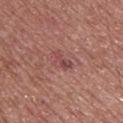Assessment: Imaged during a routine full-body skin examination; the lesion was not biopsied and no histopathology is available.